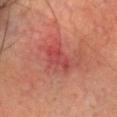The lesion was photographed on a routine skin check and not biopsied; there is no pathology result. The subject is a male aged 68–72. The lesion is on the head or neck. Automated tile analysis of the lesion measured a footprint of about 7.5 mm², an eccentricity of roughly 0.8, and two-axis asymmetry of about 0.35. And it measured an average lesion color of about L≈47 a*≈35 b*≈27 (CIELAB), roughly 7 lightness units darker than nearby skin, and a normalized border contrast of about 5.5. The lesion's longest dimension is about 4 mm. Cropped from a total-body skin-imaging series; the visible field is about 15 mm.